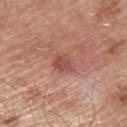Q: Was a biopsy performed?
A: no biopsy performed (imaged during a skin exam)
Q: What are the patient's age and sex?
A: male, in their mid-50s
Q: What kind of image is this?
A: ~15 mm tile from a whole-body skin photo
Q: How was the tile lit?
A: white-light
Q: Where on the body is the lesion?
A: the back
Q: How large is the lesion?
A: ~2.5 mm (longest diameter)